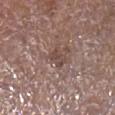Imaged during a routine full-body skin examination; the lesion was not biopsied and no histopathology is available. Imaged with white-light lighting. The recorded lesion diameter is about 2.5 mm. The lesion is on the right lower leg. A 15 mm close-up tile from a total-body photography series done for melanoma screening. A male subject in their mid- to late 70s.